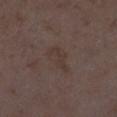No biopsy was performed on this lesion — it was imaged during a full skin examination and was not determined to be concerning. From the left lower leg. A female subject, about 35 years old. A 15 mm crop from a total-body photograph taken for skin-cancer surveillance. Longest diameter approximately 4 mm. Automated tile analysis of the lesion measured a footprint of about 7 mm² and a shape-asymmetry score of about 0.35 (0 = symmetric). It also reported a within-lesion color-variation index near 2/10 and a peripheral color-asymmetry measure near 0.5. The tile uses white-light illumination.